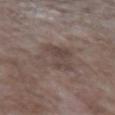imaging modality = total-body-photography crop, ~15 mm field of view | body site = the left upper arm | tile lighting = white-light illumination | patient = male, roughly 65 years of age | lesion diameter = about 4.5 mm | automated metrics = border irregularity of about 3.5 on a 0–10 scale, internal color variation of about 4 on a 0–10 scale, and a peripheral color-asymmetry measure near 1.5; an automated nevus-likeness rating near 0 out of 100.